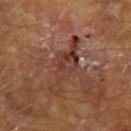Clinical impression:
The lesion was photographed on a routine skin check and not biopsied; there is no pathology result.
Image and clinical context:
On the arm. Approximately 7.5 mm at its widest. A lesion tile, about 15 mm wide, cut from a 3D total-body photograph. Automated tile analysis of the lesion measured border irregularity of about 7.5 on a 0–10 scale, a within-lesion color-variation index near 5/10, and a peripheral color-asymmetry measure near 1.5. The analysis additionally found a nevus-likeness score of about 0/100 and a detector confidence of about 10 out of 100 that the crop contains a lesion. A male subject approximately 70 years of age.